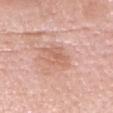Q: Was this lesion biopsied?
A: total-body-photography surveillance lesion; no biopsy
Q: What are the patient's age and sex?
A: female, roughly 70 years of age
Q: What is the imaging modality?
A: 15 mm crop, total-body photography
Q: Where on the body is the lesion?
A: the head or neck